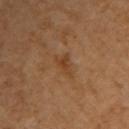Imaged during a routine full-body skin examination; the lesion was not biopsied and no histopathology is available. Captured under cross-polarized illumination. The recorded lesion diameter is about 3.5 mm. From the arm. A female subject, aged 48–52. Cropped from a total-body skin-imaging series; the visible field is about 15 mm.